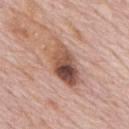{"biopsy_status": "not biopsied; imaged during a skin examination", "automated_metrics": {"eccentricity": 0.85, "shape_asymmetry": 0.2, "vs_skin_darker_L": 14.0, "vs_skin_contrast_norm": 9.5, "color_variation_0_10": 10.0, "peripheral_color_asymmetry": 5.0}, "patient": {"sex": "male", "age_approx": 75}, "site": "mid back", "image": {"source": "total-body photography crop", "field_of_view_mm": 15}, "lighting": "white-light"}